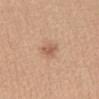Assessment:
This lesion was catalogued during total-body skin photography and was not selected for biopsy.
Background:
A region of skin cropped from a whole-body photographic capture, roughly 15 mm wide. The subject is a female aged 33 to 37. Captured under white-light illumination. On the abdomen. Longest diameter approximately 2.5 mm.